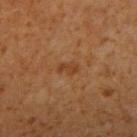<record>
<biopsy_status>not biopsied; imaged during a skin examination</biopsy_status>
<lesion_size>
  <long_diameter_mm_approx>2.5</long_diameter_mm_approx>
</lesion_size>
<lighting>cross-polarized</lighting>
<site>arm</site>
<patient>
  <sex>male</sex>
  <age_approx>60</age_approx>
</patient>
<automated_metrics>
  <lesion_detection_confidence_0_100>100</lesion_detection_confidence_0_100>
</automated_metrics>
<image>
  <source>total-body photography crop</source>
  <field_of_view_mm>15</field_of_view_mm>
</image>
</record>